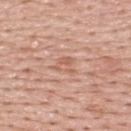workup: total-body-photography surveillance lesion; no biopsy
tile lighting: white-light
patient: male, aged 53–57
site: the upper back
acquisition: 15 mm crop, total-body photography
TBP lesion metrics: an area of roughly 4 mm², an outline eccentricity of about 0.55 (0 = round, 1 = elongated), and two-axis asymmetry of about 0.45; a lesion color around L≈60 a*≈23 b*≈30 in CIELAB, roughly 7 lightness units darker than nearby skin, and a normalized border contrast of about 5; border irregularity of about 4.5 on a 0–10 scale and internal color variation of about 3.5 on a 0–10 scale; lesion-presence confidence of about 95/100